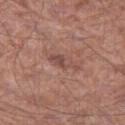No biopsy was performed on this lesion — it was imaged during a full skin examination and was not determined to be concerning. The lesion-visualizer software estimated a mean CIELAB color near L≈48 a*≈22 b*≈24 and a lesion-to-skin contrast of about 6 (normalized; higher = more distinct). It also reported a border-irregularity rating of about 4/10 and radial color variation of about 1. The software also gave an automated nevus-likeness rating near 5 out of 100 and a detector confidence of about 100 out of 100 that the crop contains a lesion. Approximately 4 mm at its widest. Located on the leg. A 15 mm close-up extracted from a 3D total-body photography capture. The tile uses white-light illumination. A male subject, about 65 years old.